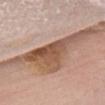No biopsy was performed on this lesion — it was imaged during a full skin examination and was not determined to be concerning. Located on the chest. The tile uses white-light illumination. Approximately 15 mm at its widest. An algorithmic analysis of the crop reported an area of roughly 28 mm², an eccentricity of roughly 0.95, and a shape-asymmetry score of about 0.65 (0 = symmetric). It also reported about 14 CIELAB-L* units darker than the surrounding skin and a normalized border contrast of about 10. And it measured a border-irregularity rating of about 10/10, a color-variation rating of about 6.5/10, and a peripheral color-asymmetry measure near 2. It also reported a nevus-likeness score of about 15/100 and a detector confidence of about 95 out of 100 that the crop contains a lesion. A female patient, aged 83 or over. Cropped from a total-body skin-imaging series; the visible field is about 15 mm.